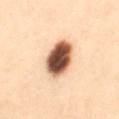The lesion was photographed on a routine skin check and not biopsied; there is no pathology result. A female patient, aged 28–32. The tile uses cross-polarized illumination. A 15 mm close-up extracted from a 3D total-body photography capture. The lesion is on the mid back. Measured at roughly 5 mm in maximum diameter.